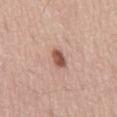{"biopsy_status": "not biopsied; imaged during a skin examination", "automated_metrics": {"area_mm2_approx": 4.0, "eccentricity": 0.75, "shape_asymmetry": 0.25}, "image": {"source": "total-body photography crop", "field_of_view_mm": 15}, "site": "mid back", "lighting": "white-light", "lesion_size": {"long_diameter_mm_approx": 2.5}, "patient": {"sex": "male", "age_approx": 60}}